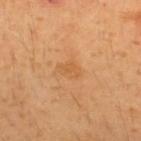Case summary:
– biopsy status — no biopsy performed (imaged during a skin exam)
– image source — ~15 mm crop, total-body skin-cancer survey
– diameter — ≈2.5 mm
– subject — male, about 30 years old
– automated lesion analysis — a lesion color around L≈56 a*≈23 b*≈42 in CIELAB and roughly 6 lightness units darker than nearby skin; a border-irregularity index near 2.5/10 and a peripheral color-asymmetry measure near 0; an automated nevus-likeness rating near 0 out of 100 and lesion-presence confidence of about 100/100
– anatomic site — the back